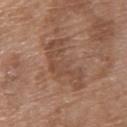biopsy status = no biopsy performed (imaged during a skin exam) | image source = total-body-photography crop, ~15 mm field of view | patient = female, aged 68 to 72 | image-analysis metrics = a footprint of about 14 mm²; a lesion color around L≈48 a*≈19 b*≈28 in CIELAB, roughly 7 lightness units darker than nearby skin, and a normalized lesion–skin contrast near 5.5; an automated nevus-likeness rating near 0 out of 100 and a detector confidence of about 100 out of 100 that the crop contains a lesion | lesion size = ≈6 mm | tile lighting = white-light illumination | anatomic site = the upper back.